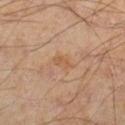Image and clinical context: A close-up tile cropped from a whole-body skin photograph, about 15 mm across. On the right lower leg. The subject is a male approximately 45 years of age.